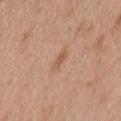No biopsy was performed on this lesion — it was imaged during a full skin examination and was not determined to be concerning.
Cropped from a total-body skin-imaging series; the visible field is about 15 mm.
The lesion is located on the upper back.
The patient is a male in their mid- to late 60s.
Automated image analysis of the tile measured a footprint of about 2.5 mm², an eccentricity of roughly 0.9, and a symmetry-axis asymmetry near 0.35. The software also gave a border-irregularity index near 4/10 and a color-variation rating of about 0/10. The software also gave a classifier nevus-likeness of about 0/100 and lesion-presence confidence of about 100/100.
Imaged with white-light lighting.
The recorded lesion diameter is about 3 mm.